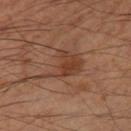Q: Was this lesion biopsied?
A: total-body-photography surveillance lesion; no biopsy
Q: How was the tile lit?
A: cross-polarized
Q: Lesion size?
A: ~6 mm (longest diameter)
Q: What did automated image analysis measure?
A: a lesion area of about 11 mm², a shape eccentricity near 0.8, and two-axis asymmetry of about 0.5; a nevus-likeness score of about 35/100 and a detector confidence of about 100 out of 100 that the crop contains a lesion
Q: What kind of image is this?
A: ~15 mm tile from a whole-body skin photo
Q: Lesion location?
A: the right thigh
Q: Who is the patient?
A: male, aged 53–57